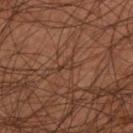notes: catalogued during a skin exam; not biopsied | patient: male, approximately 45 years of age | body site: the upper back | illumination: cross-polarized illumination | acquisition: ~15 mm crop, total-body skin-cancer survey | size: about 2 mm.